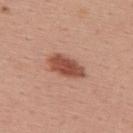Recorded during total-body skin imaging; not selected for excision or biopsy.
This is a white-light tile.
Located on the upper back.
A lesion tile, about 15 mm wide, cut from a 3D total-body photograph.
A female patient, in their mid- to late 50s.